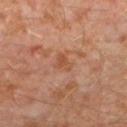Notes:
– follow-up: no biopsy performed (imaged during a skin exam)
– site: the left lower leg
– image: ~15 mm tile from a whole-body skin photo
– lesion diameter: about 2.5 mm
– image-analysis metrics: a footprint of about 3 mm² and an outline eccentricity of about 0.85 (0 = round, 1 = elongated); border irregularity of about 4 on a 0–10 scale, a color-variation rating of about 0.5/10, and a peripheral color-asymmetry measure near 0
– subject: male, aged approximately 30
– illumination: cross-polarized